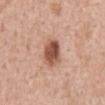Q: Was this lesion biopsied?
A: imaged on a skin check; not biopsied
Q: What is the imaging modality?
A: ~15 mm crop, total-body skin-cancer survey
Q: Lesion size?
A: about 3.5 mm
Q: What did automated image analysis measure?
A: a lesion area of about 8 mm², an eccentricity of roughly 0.7, and a symmetry-axis asymmetry near 0.15; an average lesion color of about L≈53 a*≈24 b*≈30 (CIELAB) and roughly 16 lightness units darker than nearby skin; a nevus-likeness score of about 100/100 and a detector confidence of about 100 out of 100 that the crop contains a lesion
Q: How was the tile lit?
A: white-light
Q: Lesion location?
A: the front of the torso
Q: Who is the patient?
A: male, roughly 60 years of age